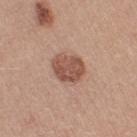{
  "biopsy_status": "not biopsied; imaged during a skin examination",
  "site": "left thigh",
  "lighting": "white-light",
  "image": {
    "source": "total-body photography crop",
    "field_of_view_mm": 15
  },
  "patient": {
    "sex": "female",
    "age_approx": 55
  },
  "lesion_size": {
    "long_diameter_mm_approx": 4.0
  }
}